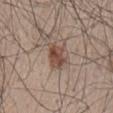* follow-up: catalogued during a skin exam; not biopsied
* imaging modality: ~15 mm crop, total-body skin-cancer survey
* site: the lower back
* patient: male, aged 63–67
* image-analysis metrics: an area of roughly 6.5 mm² and a shape eccentricity near 0.55; a lesion color around L≈47 a*≈18 b*≈25 in CIELAB and a lesion-to-skin contrast of about 8.5 (normalized; higher = more distinct); a lesion-detection confidence of about 100/100
* lesion size: about 3.5 mm